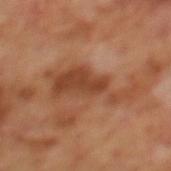Image and clinical context: Imaged with cross-polarized lighting. A male subject, aged approximately 70. A 15 mm close-up extracted from a 3D total-body photography capture. Longest diameter approximately 9 mm. From the mid back.